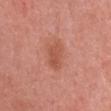<lesion>
<biopsy_status>not biopsied; imaged during a skin examination</biopsy_status>
<lesion_size>
  <long_diameter_mm_approx>3.5</long_diameter_mm_approx>
</lesion_size>
<image>
  <source>total-body photography crop</source>
  <field_of_view_mm>15</field_of_view_mm>
</image>
<automated_metrics>
  <area_mm2_approx>7.0</area_mm2_approx>
  <shape_asymmetry>0.2</shape_asymmetry>
  <cielab_L>54</cielab_L>
  <cielab_a>28</cielab_a>
  <cielab_b>32</cielab_b>
  <vs_skin_contrast_norm>6.0</vs_skin_contrast_norm>
  <border_irregularity_0_10>2.5</border_irregularity_0_10>
  <color_variation_0_10>2.0</color_variation_0_10>
  <nevus_likeness_0_100>55</nevus_likeness_0_100>
  <lesion_detection_confidence_0_100>100</lesion_detection_confidence_0_100>
</automated_metrics>
<patient>
  <sex>female</sex>
  <age_approx>35</age_approx>
</patient>
<site>chest</site>
<lighting>white-light</lighting>
</lesion>